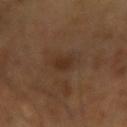Notes:
* workup: catalogued during a skin exam; not biopsied
* subject: male, roughly 65 years of age
* tile lighting: cross-polarized illumination
* automated metrics: a lesion-to-skin contrast of about 6.5 (normalized; higher = more distinct)
* image source: total-body-photography crop, ~15 mm field of view